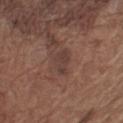Background:
This image is a 15 mm lesion crop taken from a total-body photograph. The patient is a male in their mid-70s. From the left upper arm. Captured under white-light illumination. An algorithmic analysis of the crop reported roughly 6 lightness units darker than nearby skin. And it measured border irregularity of about 4 on a 0–10 scale and a peripheral color-asymmetry measure near 0.5. The software also gave a nevus-likeness score of about 0/100 and a detector confidence of about 100 out of 100 that the crop contains a lesion.